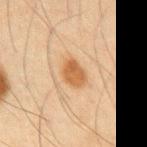<case>
<biopsy_status>not biopsied; imaged during a skin examination</biopsy_status>
<lighting>cross-polarized</lighting>
<lesion_size>
  <long_diameter_mm_approx>3.0</long_diameter_mm_approx>
</lesion_size>
<patient>
  <sex>male</sex>
  <age_approx>65</age_approx>
</patient>
<image>
  <source>total-body photography crop</source>
  <field_of_view_mm>15</field_of_view_mm>
</image>
<automated_metrics>
  <area_mm2_approx>6.0</area_mm2_approx>
  <eccentricity>0.7</eccentricity>
  <shape_asymmetry>0.2</shape_asymmetry>
  <cielab_L>49</cielab_L>
  <cielab_a>19</cielab_a>
  <cielab_b>35</cielab_b>
  <vs_skin_darker_L>10.0</vs_skin_darker_L>
  <vs_skin_contrast_norm>8.5</vs_skin_contrast_norm>
  <border_irregularity_0_10>2.0</border_irregularity_0_10>
</automated_metrics>
<site>abdomen</site>
</case>